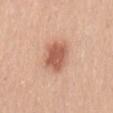follow-up: imaged on a skin check; not biopsied | image source: total-body-photography crop, ~15 mm field of view | diameter: about 4.5 mm | patient: male, about 30 years old | anatomic site: the lower back.